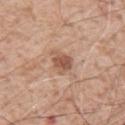<record>
  <site>left upper arm</site>
  <lesion_size>
    <long_diameter_mm_approx>3.5</long_diameter_mm_approx>
  </lesion_size>
  <patient>
    <sex>male</sex>
    <age_approx>60</age_approx>
  </patient>
  <image>
    <source>total-body photography crop</source>
    <field_of_view_mm>15</field_of_view_mm>
  </image>
</record>